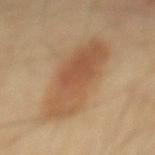- workup — total-body-photography surveillance lesion; no biopsy
- lesion size — ~9 mm (longest diameter)
- patient — male, aged 38 to 42
- illumination — cross-polarized illumination
- anatomic site — the back
- image source — 15 mm crop, total-body photography